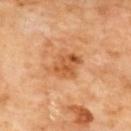The lesion was tiled from a total-body skin photograph and was not biopsied. The recorded lesion diameter is about 4 mm. The lesion is located on the back. A region of skin cropped from a whole-body photographic capture, roughly 15 mm wide. The tile uses cross-polarized illumination.